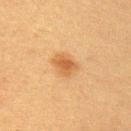patient = female, approximately 40 years of age; location = the right upper arm; acquisition = 15 mm crop, total-body photography.